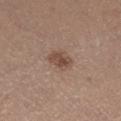Acquisition and patient details:
The recorded lesion diameter is about 3 mm. A 15 mm close-up tile from a total-body photography series done for melanoma screening. The total-body-photography lesion software estimated a classifier nevus-likeness of about 75/100. Imaged with white-light lighting. From the right lower leg. A female patient about 45 years old.